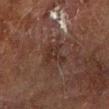{
  "biopsy_status": "not biopsied; imaged during a skin examination",
  "image": {
    "source": "total-body photography crop",
    "field_of_view_mm": 15
  },
  "patient": {
    "sex": "male",
    "age_approx": 60
  },
  "lesion_size": {
    "long_diameter_mm_approx": 3.5
  },
  "lighting": "cross-polarized",
  "site": "left forearm"
}